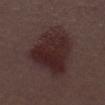{"biopsy_status": "not biopsied; imaged during a skin examination", "lighting": "white-light", "patient": {"sex": "male", "age_approx": 55}, "site": "chest", "automated_metrics": {"area_mm2_approx": 30.0, "eccentricity": 0.75, "color_variation_0_10": 4.0, "peripheral_color_asymmetry": 1.5}, "image": {"source": "total-body photography crop", "field_of_view_mm": 15}, "lesion_size": {"long_diameter_mm_approx": 8.0}}